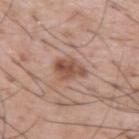Imaged during a routine full-body skin examination; the lesion was not biopsied and no histopathology is available.
A male patient, approximately 55 years of age.
Cropped from a whole-body photographic skin survey; the tile spans about 15 mm.
An algorithmic analysis of the crop reported about 11 CIELAB-L* units darker than the surrounding skin. And it measured a color-variation rating of about 4.5/10 and a peripheral color-asymmetry measure near 2. The software also gave a classifier nevus-likeness of about 80/100.
The lesion is on the left upper arm.